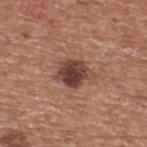biopsy status = total-body-photography surveillance lesion; no biopsy | lighting = white-light illumination | size = about 4 mm | patient = male, roughly 75 years of age | anatomic site = the upper back | imaging modality = total-body-photography crop, ~15 mm field of view.